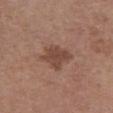Case summary:
- biopsy status: catalogued during a skin exam; not biopsied
- location: the front of the torso
- subject: female, aged 63 to 67
- image: ~15 mm tile from a whole-body skin photo
- TBP lesion metrics: an eccentricity of roughly 0.65 and two-axis asymmetry of about 0.3; an average lesion color of about L≈45 a*≈20 b*≈26 (CIELAB) and about 9 CIELAB-L* units darker than the surrounding skin
- tile lighting: white-light illumination